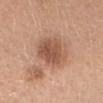The lesion was photographed on a routine skin check and not biopsied; there is no pathology result.
The subject is a female aged around 30.
The lesion is on the head or neck.
A close-up tile cropped from a whole-body skin photograph, about 15 mm across.
Imaged with white-light lighting.